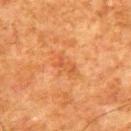Impression:
This lesion was catalogued during total-body skin photography and was not selected for biopsy.
Clinical summary:
A male patient, in their 80s. A close-up tile cropped from a whole-body skin photograph, about 15 mm across. The lesion is on the upper back. The lesion's longest dimension is about 3 mm. This is a cross-polarized tile.